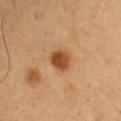Impression:
This lesion was catalogued during total-body skin photography and was not selected for biopsy.
Clinical summary:
Automated image analysis of the tile measured an eccentricity of roughly 0.45 and two-axis asymmetry of about 0.15. The software also gave a mean CIELAB color near L≈47 a*≈24 b*≈38 and a lesion–skin lightness drop of about 13. And it measured a peripheral color-asymmetry measure near 1.5. A 15 mm close-up extracted from a 3D total-body photography capture. From the left upper arm. Longest diameter approximately 3 mm. The tile uses cross-polarized illumination. The subject is a male aged 53 to 57.